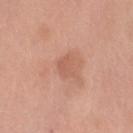{"biopsy_status": "not biopsied; imaged during a skin examination"}